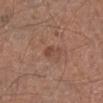notes: catalogued during a skin exam; not biopsied | image: 15 mm crop, total-body photography | body site: the left lower leg | subject: male, roughly 60 years of age | lesion diameter: about 3 mm.